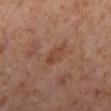{
  "lighting": "cross-polarized",
  "site": "leg",
  "patient": {
    "sex": "female",
    "age_approx": 55
  },
  "image": {
    "source": "total-body photography crop",
    "field_of_view_mm": 15
  },
  "automated_metrics": {
    "area_mm2_approx": 5.0,
    "eccentricity": 0.8,
    "color_variation_0_10": 2.5,
    "peripheral_color_asymmetry": 1.0
  }
}